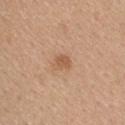Cropped from a whole-body photographic skin survey; the tile spans about 15 mm. An algorithmic analysis of the crop reported a lesion–skin lightness drop of about 9 and a lesion-to-skin contrast of about 6 (normalized; higher = more distinct). The lesion is located on the upper back. Approximately 2.5 mm at its widest. The tile uses white-light illumination. The patient is a female approximately 40 years of age.